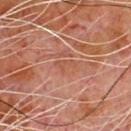This lesion was catalogued during total-body skin photography and was not selected for biopsy. Approximately 3 mm at its widest. The lesion is on the chest. A roughly 15 mm field-of-view crop from a total-body skin photograph. Automated tile analysis of the lesion measured a footprint of about 3 mm², an outline eccentricity of about 0.85 (0 = round, 1 = elongated), and a shape-asymmetry score of about 0.35 (0 = symmetric). It also reported a classifier nevus-likeness of about 0/100 and a detector confidence of about 75 out of 100 that the crop contains a lesion. The patient is a male aged approximately 80.